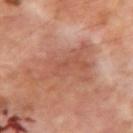follow-up: total-body-photography surveillance lesion; no biopsy | patient: male, aged 68–72 | image source: ~15 mm crop, total-body skin-cancer survey | body site: the right upper arm | image-analysis metrics: a footprint of about 20 mm² and an eccentricity of roughly 0.8; a normalized lesion–skin contrast near 5.5; a border-irregularity index near 8/10 and a within-lesion color-variation index near 2.5/10 | diameter: ~7.5 mm (longest diameter) | illumination: cross-polarized.